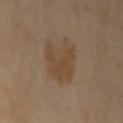Q: Is there a histopathology result?
A: no biopsy performed (imaged during a skin exam)
Q: Lesion size?
A: ~5 mm (longest diameter)
Q: What are the patient's age and sex?
A: male, in their mid- to late 60s
Q: Automated lesion metrics?
A: a lesion area of about 15 mm², a shape eccentricity near 0.65, and two-axis asymmetry of about 0.3; a mean CIELAB color near L≈44 a*≈15 b*≈30 and roughly 7 lightness units darker than nearby skin; border irregularity of about 3 on a 0–10 scale, a color-variation rating of about 2.5/10, and radial color variation of about 1; a nevus-likeness score of about 50/100
Q: How was this image acquired?
A: total-body-photography crop, ~15 mm field of view
Q: Lesion location?
A: the left upper arm
Q: Illumination type?
A: cross-polarized illumination